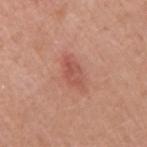The lesion was photographed on a routine skin check and not biopsied; there is no pathology result. A region of skin cropped from a whole-body photographic capture, roughly 15 mm wide. From the arm. This is a white-light tile. About 4 mm across. A male patient aged 53 to 57.